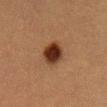Findings:
– biopsy status: total-body-photography surveillance lesion; no biopsy
– acquisition: ~15 mm crop, total-body skin-cancer survey
– patient: female, aged 53–57
– lesion size: ≈3.5 mm
– body site: the mid back